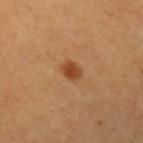Assessment: Part of a total-body skin-imaging series; this lesion was reviewed on a skin check and was not flagged for biopsy. Clinical summary: Measured at roughly 2 mm in maximum diameter. From the arm. A female subject roughly 45 years of age. Cropped from a total-body skin-imaging series; the visible field is about 15 mm. The lesion-visualizer software estimated a footprint of about 3.5 mm², an eccentricity of roughly 0.65, and two-axis asymmetry of about 0.2. The analysis additionally found an average lesion color of about L≈45 a*≈25 b*≈38 (CIELAB), about 11 CIELAB-L* units darker than the surrounding skin, and a lesion-to-skin contrast of about 9 (normalized; higher = more distinct). The analysis additionally found a border-irregularity rating of about 2/10, internal color variation of about 2 on a 0–10 scale, and radial color variation of about 0.5. It also reported a classifier nevus-likeness of about 95/100 and lesion-presence confidence of about 100/100.